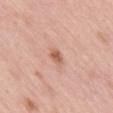Impression: Recorded during total-body skin imaging; not selected for excision or biopsy. Image and clinical context: A region of skin cropped from a whole-body photographic capture, roughly 15 mm wide. A female subject about 65 years old. Imaged with white-light lighting. Approximately 2 mm at its widest. Located on the mid back.